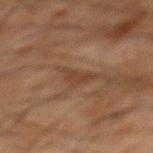Imaged during a routine full-body skin examination; the lesion was not biopsied and no histopathology is available.
On the back.
This image is a 15 mm lesion crop taken from a total-body photograph.
Imaged with cross-polarized lighting.
About 3 mm across.
The subject is a male aged 63–67.